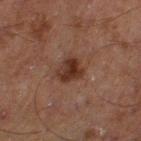Q: Was a biopsy performed?
A: no biopsy performed (imaged during a skin exam)
Q: What is the lesion's diameter?
A: ≈3 mm
Q: What are the patient's age and sex?
A: male, approximately 70 years of age
Q: What is the imaging modality?
A: ~15 mm crop, total-body skin-cancer survey
Q: Lesion location?
A: the left thigh
Q: What did automated image analysis measure?
A: a footprint of about 6.5 mm², an eccentricity of roughly 0.4, and two-axis asymmetry of about 0.3; an average lesion color of about L≈26 a*≈17 b*≈22 (CIELAB), about 9 CIELAB-L* units darker than the surrounding skin, and a normalized border contrast of about 10; border irregularity of about 3 on a 0–10 scale, a within-lesion color-variation index near 5/10, and radial color variation of about 1.5